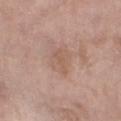Clinical impression: The lesion was tiled from a total-body skin photograph and was not biopsied. Background: A female patient, roughly 75 years of age. A 15 mm crop from a total-body photograph taken for skin-cancer surveillance. Approximately 3 mm at its widest. On the leg.